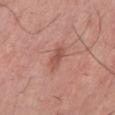biopsy_status: not biopsied; imaged during a skin examination
patient:
  sex: female
  age_approx: 40
automated_metrics:
  color_variation_0_10: 2.0
  peripheral_color_asymmetry: 1.0
lighting: white-light
site: left lower leg
image:
  source: total-body photography crop
  field_of_view_mm: 15
lesion_size:
  long_diameter_mm_approx: 3.0